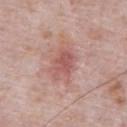Impression:
Captured during whole-body skin photography for melanoma surveillance; the lesion was not biopsied.
Clinical summary:
Approximately 4 mm at its widest. A male patient, aged around 70. The lesion is located on the abdomen. A region of skin cropped from a whole-body photographic capture, roughly 15 mm wide.